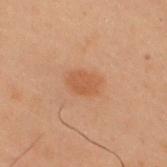The lesion was tiled from a total-body skin photograph and was not biopsied.
On the right upper arm.
The total-body-photography lesion software estimated a lesion area of about 6 mm², an eccentricity of roughly 0.7, and two-axis asymmetry of about 0.2. The software also gave a border-irregularity index near 2/10, a within-lesion color-variation index near 1.5/10, and peripheral color asymmetry of about 0.5. And it measured an automated nevus-likeness rating near 75 out of 100.
A 15 mm crop from a total-body photograph taken for skin-cancer surveillance.
The patient is a male approximately 55 years of age.
This is a cross-polarized tile.